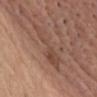patient:
  sex: male
  age_approx: 60
image:
  source: total-body photography crop
  field_of_view_mm: 15
site: head or neck
lesion_size:
  long_diameter_mm_approx: 6.0